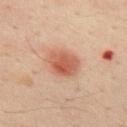The lesion was photographed on a routine skin check and not biopsied; there is no pathology result. A region of skin cropped from a whole-body photographic capture, roughly 15 mm wide. The lesion's longest dimension is about 4 mm. The lesion is on the back. This is a cross-polarized tile. A male patient, in their 50s.